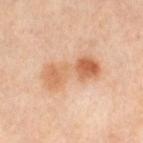Recorded during total-body skin imaging; not selected for excision or biopsy.
This image is a 15 mm lesion crop taken from a total-body photograph.
The tile uses cross-polarized illumination.
From the leg.
A female patient aged approximately 50.